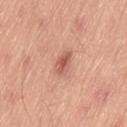Notes:
• workup — imaged on a skin check; not biopsied
• image-analysis metrics — a mean CIELAB color near L≈58 a*≈26 b*≈30, roughly 11 lightness units darker than nearby skin, and a normalized lesion–skin contrast near 7; an automated nevus-likeness rating near 80 out of 100 and lesion-presence confidence of about 100/100
• imaging modality — ~15 mm crop, total-body skin-cancer survey
• site — the left thigh
• subject — male, approximately 50 years of age
• illumination — white-light illumination
• diameter — ~3 mm (longest diameter)